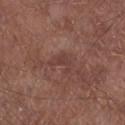workup — no biopsy performed (imaged during a skin exam)
lesion size — ≈3 mm
tile lighting — white-light
subject — male, about 55 years old
body site — the left lower leg
imaging modality — total-body-photography crop, ~15 mm field of view